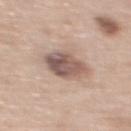Assessment:
Captured during whole-body skin photography for melanoma surveillance; the lesion was not biopsied.
Image and clinical context:
A female subject approximately 60 years of age. The lesion's longest dimension is about 4 mm. The total-body-photography lesion software estimated a color-variation rating of about 6.5/10. It also reported a lesion-detection confidence of about 100/100. On the upper back. This is a white-light tile. A lesion tile, about 15 mm wide, cut from a 3D total-body photograph.